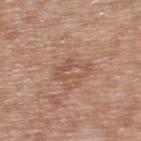Notes:
- notes · total-body-photography surveillance lesion; no biopsy